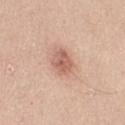| field | value |
|---|---|
| biopsy status | total-body-photography surveillance lesion; no biopsy |
| patient | female, aged 38 to 42 |
| site | the left thigh |
| tile lighting | white-light illumination |
| acquisition | ~15 mm crop, total-body skin-cancer survey |
| size | ~3 mm (longest diameter) |
| automated metrics | a lesion color around L≈60 a*≈23 b*≈29 in CIELAB and about 11 CIELAB-L* units darker than the surrounding skin |